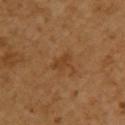biopsy status: total-body-photography surveillance lesion; no biopsy
patient: female, in their mid- to late 50s
size: ≈2.5 mm
tile lighting: cross-polarized illumination
site: the upper back
image source: 15 mm crop, total-body photography